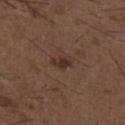Clinical impression:
Captured during whole-body skin photography for melanoma surveillance; the lesion was not biopsied.
Background:
Longest diameter approximately 3 mm. A 15 mm close-up extracted from a 3D total-body photography capture. Automated image analysis of the tile measured a border-irregularity rating of about 3/10 and internal color variation of about 3 on a 0–10 scale. The subject is a male approximately 50 years of age. The tile uses white-light illumination. Located on the upper back.